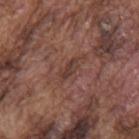On the mid back.
A male patient aged approximately 75.
A 15 mm close-up extracted from a 3D total-body photography capture.